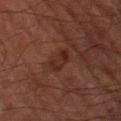This lesion was catalogued during total-body skin photography and was not selected for biopsy.
An algorithmic analysis of the crop reported a footprint of about 4.5 mm², a shape eccentricity near 0.75, and a symmetry-axis asymmetry near 0.3.
Measured at roughly 2.5 mm in maximum diameter.
Cropped from a whole-body photographic skin survey; the tile spans about 15 mm.
A male subject, aged around 65.
On the right thigh.